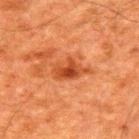Q: Is there a histopathology result?
A: catalogued during a skin exam; not biopsied
Q: Illumination type?
A: cross-polarized
Q: Where on the body is the lesion?
A: the upper back
Q: Lesion size?
A: about 4 mm
Q: Automated lesion metrics?
A: border irregularity of about 3.5 on a 0–10 scale, a within-lesion color-variation index near 4.5/10, and radial color variation of about 1.5; a nevus-likeness score of about 70/100 and a lesion-detection confidence of about 100/100
Q: What kind of image is this?
A: ~15 mm crop, total-body skin-cancer survey
Q: What are the patient's age and sex?
A: male, in their 60s